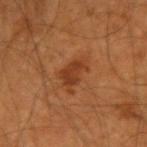<lesion>
<biopsy_status>not biopsied; imaged during a skin examination</biopsy_status>
<site>right forearm</site>
<lesion_size>
  <long_diameter_mm_approx>4.0</long_diameter_mm_approx>
</lesion_size>
<patient>
  <sex>male</sex>
  <age_approx>55</age_approx>
</patient>
<image>
  <source>total-body photography crop</source>
  <field_of_view_mm>15</field_of_view_mm>
</image>
<automated_metrics>
  <area_mm2_approx>7.5</area_mm2_approx>
  <eccentricity>0.75</eccentricity>
  <shape_asymmetry>0.4</shape_asymmetry>
  <border_irregularity_0_10>4.5</border_irregularity_0_10>
  <color_variation_0_10>2.0</color_variation_0_10>
  <peripheral_color_asymmetry>0.5</peripheral_color_asymmetry>
  <nevus_likeness_0_100>75</nevus_likeness_0_100>
  <lesion_detection_confidence_0_100>100</lesion_detection_confidence_0_100>
</automated_metrics>
</lesion>